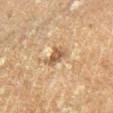Clinical impression: Recorded during total-body skin imaging; not selected for excision or biopsy. Image and clinical context: A lesion tile, about 15 mm wide, cut from a 3D total-body photograph. A male subject aged 58–62. The lesion is on the right lower leg. The lesion-visualizer software estimated a footprint of about 4.5 mm², an outline eccentricity of about 0.75 (0 = round, 1 = elongated), and a shape-asymmetry score of about 0.25 (0 = symmetric). It also reported roughly 9 lightness units darker than nearby skin and a lesion-to-skin contrast of about 8 (normalized; higher = more distinct). The software also gave a within-lesion color-variation index near 2.5/10. The lesion's longest dimension is about 3 mm. Imaged with cross-polarized lighting.